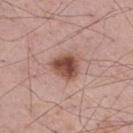A male patient, about 55 years old.
On the right thigh.
Automated image analysis of the tile measured a color-variation rating of about 5.5/10 and a peripheral color-asymmetry measure near 2. The software also gave a classifier nevus-likeness of about 90/100.
Longest diameter approximately 3.5 mm.
This is a white-light tile.
A 15 mm crop from a total-body photograph taken for skin-cancer surveillance.